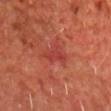Case summary:
* notes — catalogued during a skin exam; not biopsied
* tile lighting — cross-polarized
* image source — 15 mm crop, total-body photography
* patient — male, roughly 70 years of age
* body site — the front of the torso
* diameter — ≈3 mm